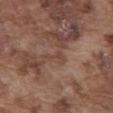The lesion was photographed on a routine skin check and not biopsied; there is no pathology result. A lesion tile, about 15 mm wide, cut from a 3D total-body photograph. The recorded lesion diameter is about 3 mm. Imaged with white-light lighting. A male patient, aged approximately 75. The lesion is located on the abdomen.